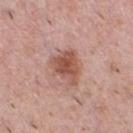- biopsy status — catalogued during a skin exam; not biopsied
- subject — male, approximately 40 years of age
- site — the chest
- automated lesion analysis — a nevus-likeness score of about 85/100 and a detector confidence of about 100 out of 100 that the crop contains a lesion
- acquisition — ~15 mm crop, total-body skin-cancer survey
- tile lighting — white-light